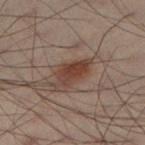<tbp_lesion>
<biopsy_status>not biopsied; imaged during a skin examination</biopsy_status>
<patient>
  <sex>male</sex>
  <age_approx>50</age_approx>
</patient>
<site>left lower leg</site>
<image>
  <source>total-body photography crop</source>
  <field_of_view_mm>15</field_of_view_mm>
</image>
<lesion_size>
  <long_diameter_mm_approx>4.0</long_diameter_mm_approx>
</lesion_size>
<lighting>cross-polarized</lighting>
</tbp_lesion>